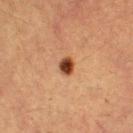Case summary:
– workup · catalogued during a skin exam; not biopsied
– body site · the right lower leg
– illumination · cross-polarized
– diameter · ≈2.5 mm
– automated metrics · an area of roughly 4 mm², a shape eccentricity near 0.6, and a shape-asymmetry score of about 0.3 (0 = symmetric); a lesion color around L≈36 a*≈21 b*≈29 in CIELAB and about 15 CIELAB-L* units darker than the surrounding skin; a detector confidence of about 100 out of 100 that the crop contains a lesion
– acquisition · 15 mm crop, total-body photography
– subject · female, aged approximately 55